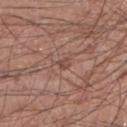Impression: The lesion was tiled from a total-body skin photograph and was not biopsied. Context: A male patient, in their mid- to late 40s. A 15 mm close-up extracted from a 3D total-body photography capture. The lesion is on the right lower leg.